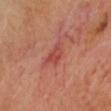The lesion was photographed on a routine skin check and not biopsied; there is no pathology result. On the head or neck. This is a cross-polarized tile. The lesion-visualizer software estimated an area of roughly 3.5 mm² and a shape-asymmetry score of about 0.45 (0 = symmetric). The software also gave a detector confidence of about 100 out of 100 that the crop contains a lesion. A close-up tile cropped from a whole-body skin photograph, about 15 mm across.